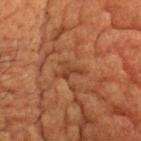  biopsy_status: not biopsied; imaged during a skin examination
  patient:
    sex: female
    age_approx: 80
  site: head or neck
  lighting: cross-polarized
  lesion_size:
    long_diameter_mm_approx: 3.0
  image:
    source: total-body photography crop
    field_of_view_mm: 15